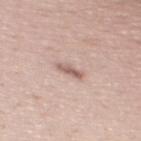Notes:
- follow-up — imaged on a skin check; not biopsied
- subject — male, aged 53–57
- acquisition — ~15 mm tile from a whole-body skin photo
- TBP lesion metrics — a footprint of about 3.5 mm² and an eccentricity of roughly 0.95; an average lesion color of about L≈61 a*≈18 b*≈24 (CIELAB), about 11 CIELAB-L* units darker than the surrounding skin, and a lesion-to-skin contrast of about 7 (normalized; higher = more distinct); a within-lesion color-variation index near 1/10 and peripheral color asymmetry of about 0
- lesion size — ≈3.5 mm
- anatomic site — the mid back